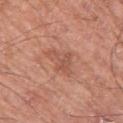Imaged during a routine full-body skin examination; the lesion was not biopsied and no histopathology is available. A 15 mm crop from a total-body photograph taken for skin-cancer surveillance. The lesion is on the left thigh. A male patient aged 58 to 62. An algorithmic analysis of the crop reported a lesion-to-skin contrast of about 5 (normalized; higher = more distinct). The software also gave an automated nevus-likeness rating near 0 out of 100 and a lesion-detection confidence of about 100/100. Imaged with white-light lighting. Measured at roughly 3.5 mm in maximum diameter.